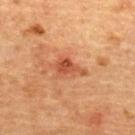Clinical impression:
Part of a total-body skin-imaging series; this lesion was reviewed on a skin check and was not flagged for biopsy.
Background:
On the upper back. A female subject approximately 70 years of age. This image is a 15 mm lesion crop taken from a total-body photograph.